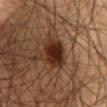workup: total-body-photography surveillance lesion; no biopsy
tile lighting: cross-polarized illumination
size: ≈4.5 mm
image source: ~15 mm crop, total-body skin-cancer survey
automated metrics: an eccentricity of roughly 0.75 and two-axis asymmetry of about 0.25; roughly 12 lightness units darker than nearby skin; a classifier nevus-likeness of about 95/100
patient: male, in their 50s
site: the abdomen